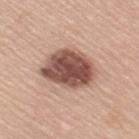Clinical impression:
No biopsy was performed on this lesion — it was imaged during a full skin examination and was not determined to be concerning.
Image and clinical context:
The lesion is located on the right upper arm. An algorithmic analysis of the crop reported a mean CIELAB color near L≈51 a*≈21 b*≈25, a lesion–skin lightness drop of about 18, and a normalized border contrast of about 12. It also reported a border-irregularity index near 2/10, internal color variation of about 6 on a 0–10 scale, and peripheral color asymmetry of about 2. Measured at roughly 6.5 mm in maximum diameter. A 15 mm close-up tile from a total-body photography series done for melanoma screening. The tile uses white-light illumination. A female subject aged around 60.